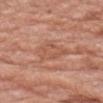• size: ~3.5 mm (longest diameter)
• TBP lesion metrics: a border-irregularity index near 3.5/10
• patient: female, about 75 years old
• anatomic site: the left forearm
• illumination: white-light
• image: 15 mm crop, total-body photography